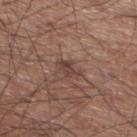{"biopsy_status": "not biopsied; imaged during a skin examination", "patient": {"sex": "male", "age_approx": 60}, "automated_metrics": {"border_irregularity_0_10": 3.5, "color_variation_0_10": 3.0, "peripheral_color_asymmetry": 1.0, "nevus_likeness_0_100": 0, "lesion_detection_confidence_0_100": 95}, "lighting": "white-light", "image": {"source": "total-body photography crop", "field_of_view_mm": 15}, "site": "left lower leg", "lesion_size": {"long_diameter_mm_approx": 2.5}}